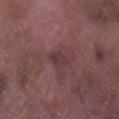Captured during whole-body skin photography for melanoma surveillance; the lesion was not biopsied. Located on the right lower leg. Cropped from a whole-body photographic skin survey; the tile spans about 15 mm. Longest diameter approximately 2.5 mm. The subject is a male aged 73 to 77. This is a white-light tile.